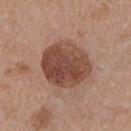Findings:
* biopsy status · total-body-photography surveillance lesion; no biopsy
* patient · female, aged approximately 35
* automated metrics · a footprint of about 25 mm² and an outline eccentricity of about 0.45 (0 = round, 1 = elongated); about 13 CIELAB-L* units darker than the surrounding skin and a lesion-to-skin contrast of about 9.5 (normalized; higher = more distinct); internal color variation of about 5 on a 0–10 scale and radial color variation of about 1.5
* anatomic site · the chest
* lesion size · ≈6 mm
* lighting · white-light illumination
* image · total-body-photography crop, ~15 mm field of view